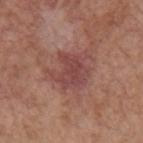Captured during whole-body skin photography for melanoma surveillance; the lesion was not biopsied.
Automated image analysis of the tile measured a footprint of about 13 mm², an outline eccentricity of about 0.45 (0 = round, 1 = elongated), and two-axis asymmetry of about 0.4. The software also gave a mean CIELAB color near L≈45 a*≈25 b*≈23 and about 8 CIELAB-L* units darker than the surrounding skin. The software also gave a nevus-likeness score of about 0/100 and lesion-presence confidence of about 100/100.
The lesion's longest dimension is about 4.5 mm.
Located on the left upper arm.
The patient is a female aged 63–67.
This is a white-light tile.
A region of skin cropped from a whole-body photographic capture, roughly 15 mm wide.